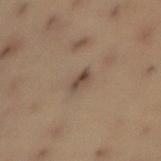biopsy status: catalogued during a skin exam; not biopsied | lighting: cross-polarized illumination | site: the front of the torso | patient: male, aged 53 to 57 | acquisition: 15 mm crop, total-body photography | TBP lesion metrics: a footprint of about 2.5 mm², an outline eccentricity of about 0.9 (0 = round, 1 = elongated), and a shape-asymmetry score of about 0.3 (0 = symmetric); a mean CIELAB color near L≈43 a*≈14 b*≈23, roughly 10 lightness units darker than nearby skin, and a lesion-to-skin contrast of about 8.5 (normalized; higher = more distinct); an automated nevus-likeness rating near 25 out of 100 and lesion-presence confidence of about 95/100 | diameter: ≈3 mm.